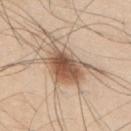follow-up: no biopsy performed (imaged during a skin exam) | patient: male, aged around 45 | image source: total-body-photography crop, ~15 mm field of view | location: the upper back.